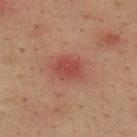<case>
<biopsy_status>not biopsied; imaged during a skin examination</biopsy_status>
<automated_metrics>
  <nevus_likeness_0_100>0</nevus_likeness_0_100>
  <lesion_detection_confidence_0_100>100</lesion_detection_confidence_0_100>
</automated_metrics>
<image>
  <source>total-body photography crop</source>
  <field_of_view_mm>15</field_of_view_mm>
</image>
<lighting>cross-polarized</lighting>
<site>upper back</site>
<patient>
  <sex>male</sex>
  <age_approx>35</age_approx>
</patient>
</case>